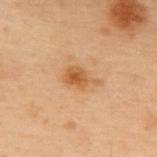Captured during whole-body skin photography for melanoma surveillance; the lesion was not biopsied. A male patient in their mid-50s. Cropped from a total-body skin-imaging series; the visible field is about 15 mm. About 3.5 mm across. The tile uses cross-polarized illumination. Located on the upper back.